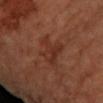Clinical impression:
The lesion was tiled from a total-body skin photograph and was not biopsied.
Image and clinical context:
The subject is a female approximately 70 years of age. A roughly 15 mm field-of-view crop from a total-body skin photograph. The lesion is on the left forearm.